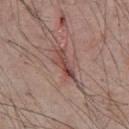Q: Is there a histopathology result?
A: total-body-photography surveillance lesion; no biopsy